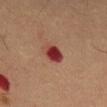| feature | finding |
|---|---|
| biopsy status | total-body-photography surveillance lesion; no biopsy |
| lighting | cross-polarized illumination |
| body site | the mid back |
| image | ~15 mm crop, total-body skin-cancer survey |
| subject | male, in their mid- to late 50s |